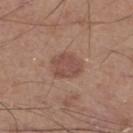No biopsy was performed on this lesion — it was imaged during a full skin examination and was not determined to be concerning.
A region of skin cropped from a whole-body photographic capture, roughly 15 mm wide.
The lesion is located on the right lower leg.
About 3.5 mm across.
A male subject, aged approximately 60.
Imaged with white-light lighting.